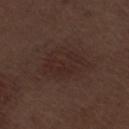Impression: The lesion was photographed on a routine skin check and not biopsied; there is no pathology result. Acquisition and patient details: The subject is a male aged around 70. The lesion's longest dimension is about 6 mm. A roughly 15 mm field-of-view crop from a total-body skin photograph. From the right thigh. The tile uses white-light illumination.